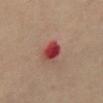notes: total-body-photography surveillance lesion; no biopsy | tile lighting: cross-polarized illumination | automated metrics: a footprint of about 6 mm² and an outline eccentricity of about 0.45 (0 = round, 1 = elongated); an average lesion color of about L≈41 a*≈34 b*≈25 (CIELAB); border irregularity of about 1.5 on a 0–10 scale and peripheral color asymmetry of about 1.5; lesion-presence confidence of about 100/100 | lesion diameter: about 3 mm | imaging modality: ~15 mm crop, total-body skin-cancer survey | anatomic site: the abdomen | subject: female, in their mid-60s.